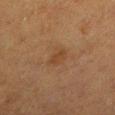workup — no biopsy performed (imaged during a skin exam) | patient — female, in their 40s | body site — the left lower leg | lesion size — ~2.5 mm (longest diameter) | acquisition — ~15 mm tile from a whole-body skin photo | lighting — cross-polarized illumination.